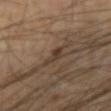Impression:
The lesion was tiled from a total-body skin photograph and was not biopsied.